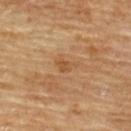Impression:
Captured during whole-body skin photography for melanoma surveillance; the lesion was not biopsied.
Context:
The lesion is located on the back. A 15 mm close-up extracted from a 3D total-body photography capture. The subject is a male aged 83 or older.